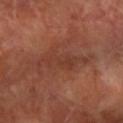No biopsy was performed on this lesion — it was imaged during a full skin examination and was not determined to be concerning. A female patient approximately 60 years of age. Longest diameter approximately 6.5 mm. Located on the left arm. The total-body-photography lesion software estimated an area of roughly 9 mm², an eccentricity of roughly 0.95, and a shape-asymmetry score of about 0.4 (0 = symmetric). And it measured border irregularity of about 7 on a 0–10 scale, internal color variation of about 2.5 on a 0–10 scale, and a peripheral color-asymmetry measure near 1. It also reported an automated nevus-likeness rating near 0 out of 100. A lesion tile, about 15 mm wide, cut from a 3D total-body photograph.